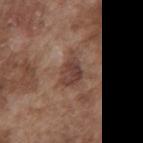Notes:
* notes · catalogued during a skin exam; not biopsied
* acquisition · 15 mm crop, total-body photography
* anatomic site · the front of the torso
* subject · male, about 75 years old